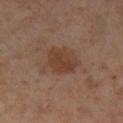follow-up: imaged on a skin check; not biopsied
patient: female, in their mid- to late 50s
lesion diameter: about 4.5 mm
imaging modality: 15 mm crop, total-body photography
tile lighting: cross-polarized illumination
body site: the left lower leg